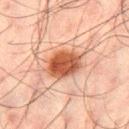notes — total-body-photography surveillance lesion; no biopsy | anatomic site — the right thigh | tile lighting — cross-polarized illumination | patient — male, aged approximately 50 | automated metrics — an area of roughly 12 mm² and a shape-asymmetry score of about 0.1 (0 = symmetric) | imaging modality — ~15 mm crop, total-body skin-cancer survey.